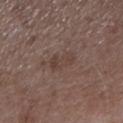The subject is a male in their 50s. Located on the arm. A 15 mm close-up extracted from a 3D total-body photography capture.